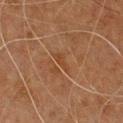The lesion was photographed on a routine skin check and not biopsied; there is no pathology result. Imaged with cross-polarized lighting. A male subject roughly 65 years of age. On the front of the torso. A lesion tile, about 15 mm wide, cut from a 3D total-body photograph. Approximately 2.5 mm at its widest.